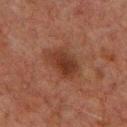workup — catalogued during a skin exam; not biopsied
automated lesion analysis — a footprint of about 9.5 mm², an outline eccentricity of about 0.75 (0 = round, 1 = elongated), and a symmetry-axis asymmetry near 0.25; a mean CIELAB color near L≈27 a*≈18 b*≈24, roughly 7 lightness units darker than nearby skin, and a lesion-to-skin contrast of about 8 (normalized; higher = more distinct)
site — the chest
patient — male, approximately 60 years of age
image source — ~15 mm crop, total-body skin-cancer survey